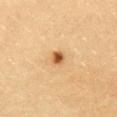Case summary:
• body site · the chest
• imaging modality · 15 mm crop, total-body photography
• patient · female, aged 18 to 22